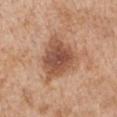<record>
  <biopsy_status>not biopsied; imaged during a skin examination</biopsy_status>
  <lighting>white-light</lighting>
  <site>left upper arm</site>
  <image>
    <source>total-body photography crop</source>
    <field_of_view_mm>15</field_of_view_mm>
  </image>
  <patient>
    <sex>male</sex>
    <age_approx>65</age_approx>
  </patient>
  <lesion_size>
    <long_diameter_mm_approx>5.5</long_diameter_mm_approx>
  </lesion_size>
  <automated_metrics>
    <area_mm2_approx>16.0</area_mm2_approx>
    <eccentricity>0.5</eccentricity>
    <shape_asymmetry>0.3</shape_asymmetry>
    <cielab_L>52</cielab_L>
    <cielab_a>22</cielab_a>
    <cielab_b>31</cielab_b>
    <vs_skin_darker_L>13.0</vs_skin_darker_L>
  </automated_metrics>
</record>